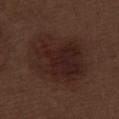Assessment: Part of a total-body skin-imaging series; this lesion was reviewed on a skin check and was not flagged for biopsy. Context: The tile uses white-light illumination. The patient is a male approximately 70 years of age. Measured at roughly 8.5 mm in maximum diameter. A 15 mm close-up extracted from a 3D total-body photography capture. The lesion-visualizer software estimated border irregularity of about 2.5 on a 0–10 scale and radial color variation of about 1.5. From the left thigh.